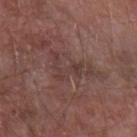  biopsy_status: not biopsied; imaged during a skin examination
  site: left forearm
  patient:
    sex: male
    age_approx: 80
  automated_metrics:
    area_mm2_approx: 8.5
    eccentricity: 0.85
    cielab_L: 38
    cielab_a: 19
    cielab_b: 20
    vs_skin_darker_L: 6.0
    color_variation_0_10: 2.5
    peripheral_color_asymmetry: 1.0
  lesion_size:
    long_diameter_mm_approx: 5.5
  image:
    source: total-body photography crop
    field_of_view_mm: 15
  lighting: white-light